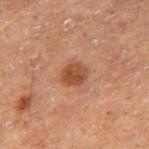The lesion was photographed on a routine skin check and not biopsied; there is no pathology result. A male subject, roughly 70 years of age. On the right thigh. A lesion tile, about 15 mm wide, cut from a 3D total-body photograph. The total-body-photography lesion software estimated a lesion area of about 6 mm² and an outline eccentricity of about 0.55 (0 = round, 1 = elongated). The analysis additionally found a nevus-likeness score of about 85/100 and a detector confidence of about 100 out of 100 that the crop contains a lesion. The tile uses cross-polarized illumination.